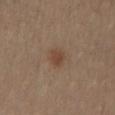Image and clinical context:
Measured at roughly 3 mm in maximum diameter. A close-up tile cropped from a whole-body skin photograph, about 15 mm across. Located on the right leg. The patient is a female aged 38 to 42.